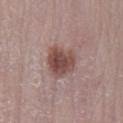Captured during whole-body skin photography for melanoma surveillance; the lesion was not biopsied.
A female patient aged around 40.
Automated image analysis of the tile measured a footprint of about 11 mm², an outline eccentricity of about 0.5 (0 = round, 1 = elongated), and a symmetry-axis asymmetry near 0.25. And it measured a lesion color around L≈48 a*≈20 b*≈21 in CIELAB and a lesion-to-skin contrast of about 9 (normalized; higher = more distinct). The analysis additionally found border irregularity of about 2.5 on a 0–10 scale, internal color variation of about 4.5 on a 0–10 scale, and a peripheral color-asymmetry measure near 1.5. The software also gave lesion-presence confidence of about 100/100.
Approximately 4 mm at its widest.
A region of skin cropped from a whole-body photographic capture, roughly 15 mm wide.
From the left lower leg.